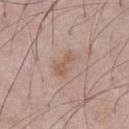{"biopsy_status": "not biopsied; imaged during a skin examination", "patient": {"sex": "male", "age_approx": 65}, "lesion_size": {"long_diameter_mm_approx": 3.5}, "lighting": "white-light", "automated_metrics": {"cielab_L": 57, "cielab_a": 18, "cielab_b": 27, "vs_skin_contrast_norm": 6.0, "lesion_detection_confidence_0_100": 100}, "image": {"source": "total-body photography crop", "field_of_view_mm": 15}, "site": "chest"}